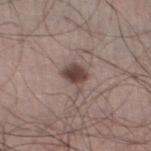Case summary:
• workup · catalogued during a skin exam; not biopsied
• anatomic site · the left thigh
• automated metrics · an area of roughly 5.5 mm² and a shape eccentricity near 0.6; border irregularity of about 3 on a 0–10 scale and a peripheral color-asymmetry measure near 1
• lighting · white-light
• subject · male, about 35 years old
• image source · total-body-photography crop, ~15 mm field of view
• size · ~3 mm (longest diameter)